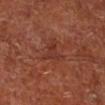Impression:
This lesion was catalogued during total-body skin photography and was not selected for biopsy.
Acquisition and patient details:
This image is a 15 mm lesion crop taken from a total-body photograph. The lesion is on the leg. A male subject aged approximately 65. Captured under cross-polarized illumination. Approximately 3.5 mm at its widest.